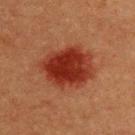Notes:
• notes · catalogued during a skin exam; not biopsied
• image source · ~15 mm crop, total-body skin-cancer survey
• lighting · cross-polarized illumination
• lesion diameter · ~6 mm (longest diameter)
• image-analysis metrics · a lesion color around L≈29 a*≈28 b*≈29 in CIELAB and about 12 CIELAB-L* units darker than the surrounding skin; a nevus-likeness score of about 100/100
• location · the upper back
• subject · male, roughly 40 years of age